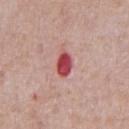Captured during whole-body skin photography for melanoma surveillance; the lesion was not biopsied. Imaged with white-light lighting. On the abdomen. Longest diameter approximately 3.5 mm. A 15 mm close-up tile from a total-body photography series done for melanoma screening. The subject is a male aged approximately 60.